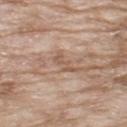About 3 mm across.
A 15 mm crop from a total-body photograph taken for skin-cancer surveillance.
A male subject aged 63–67.
An algorithmic analysis of the crop reported a lesion color around L≈56 a*≈17 b*≈29 in CIELAB, roughly 8 lightness units darker than nearby skin, and a normalized lesion–skin contrast near 5.5. And it measured a border-irregularity index near 6.5/10, internal color variation of about 0 on a 0–10 scale, and peripheral color asymmetry of about 0.
On the upper back.
This is a white-light tile.